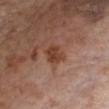No biopsy was performed on this lesion — it was imaged during a full skin examination and was not determined to be concerning. The recorded lesion diameter is about 3 mm. A female patient, in their mid-80s or older. Cropped from a total-body skin-imaging series; the visible field is about 15 mm. On the chest.